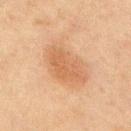Notes:
– biopsy status — catalogued during a skin exam; not biopsied
– automated metrics — an average lesion color of about L≈50 a*≈18 b*≈31 (CIELAB), about 7 CIELAB-L* units darker than the surrounding skin, and a normalized border contrast of about 6; a nevus-likeness score of about 65/100
– anatomic site — the chest
– subject — male, aged around 65
– size — ≈6 mm
– imaging modality — 15 mm crop, total-body photography